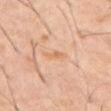Q: Was a biopsy performed?
A: total-body-photography surveillance lesion; no biopsy
Q: What kind of image is this?
A: ~15 mm crop, total-body skin-cancer survey
Q: Patient demographics?
A: male, aged 58–62
Q: What is the anatomic site?
A: the abdomen